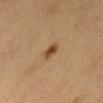{
  "biopsy_status": "not biopsied; imaged during a skin examination",
  "image": {
    "source": "total-body photography crop",
    "field_of_view_mm": 15
  },
  "patient": {
    "sex": "male",
    "age_approx": 60
  },
  "automated_metrics": {
    "cielab_L": 46,
    "cielab_a": 20,
    "cielab_b": 36,
    "vs_skin_darker_L": 12.0,
    "vs_skin_contrast_norm": 9.5,
    "border_irregularity_0_10": 3.0,
    "color_variation_0_10": 3.0
  },
  "site": "abdomen"
}